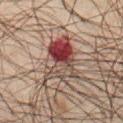Clinical impression: No biopsy was performed on this lesion — it was imaged during a full skin examination and was not determined to be concerning. Context: The tile uses cross-polarized illumination. Longest diameter approximately 6.5 mm. Located on the front of the torso. This image is a 15 mm lesion crop taken from a total-body photograph. A male subject, roughly 65 years of age.